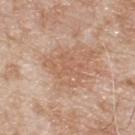The lesion was tiled from a total-body skin photograph and was not biopsied. A 15 mm close-up tile from a total-body photography series done for melanoma screening. A male patient, in their 80s. Located on the upper back. This is a white-light tile. The total-body-photography lesion software estimated a mean CIELAB color near L≈59 a*≈20 b*≈31, a lesion–skin lightness drop of about 7, and a normalized border contrast of about 5. The analysis additionally found a border-irregularity index near 8/10 and a peripheral color-asymmetry measure near 0.5. The analysis additionally found a nevus-likeness score of about 0/100 and lesion-presence confidence of about 100/100.